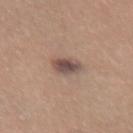<record>
<biopsy_status>not biopsied; imaged during a skin examination</biopsy_status>
<image>
  <source>total-body photography crop</source>
  <field_of_view_mm>15</field_of_view_mm>
</image>
<lesion_size>
  <long_diameter_mm_approx>2.5</long_diameter_mm_approx>
</lesion_size>
<site>front of the torso</site>
<patient>
  <sex>female</sex>
  <age_approx>55</age_approx>
</patient>
</record>